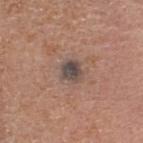{
  "biopsy_status": "not biopsied; imaged during a skin examination",
  "lesion_size": {
    "long_diameter_mm_approx": 2.5
  },
  "site": "head or neck",
  "lighting": "white-light",
  "patient": {
    "sex": "female",
    "age_approx": 75
  },
  "image": {
    "source": "total-body photography crop",
    "field_of_view_mm": 15
  },
  "automated_metrics": {
    "cielab_L": 46,
    "cielab_a": 12,
    "cielab_b": 19,
    "vs_skin_darker_L": 11.0,
    "vs_skin_contrast_norm": 10.0,
    "border_irregularity_0_10": 2.0,
    "peripheral_color_asymmetry": 1.5,
    "nevus_likeness_0_100": 5
  }
}